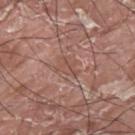{
  "biopsy_status": "not biopsied; imaged during a skin examination",
  "image": {
    "source": "total-body photography crop",
    "field_of_view_mm": 15
  },
  "site": "upper back",
  "lesion_size": {
    "long_diameter_mm_approx": 2.5
  },
  "lighting": "white-light",
  "automated_metrics": {
    "cielab_L": 50,
    "cielab_a": 21,
    "cielab_b": 26,
    "vs_skin_darker_L": 6.0,
    "vs_skin_contrast_norm": 5.0,
    "border_irregularity_0_10": 5.0,
    "color_variation_0_10": 2.0,
    "peripheral_color_asymmetry": 1.0
  },
  "patient": {
    "sex": "male",
    "age_approx": 40
  }
}